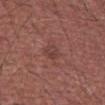The lesion was photographed on a routine skin check and not biopsied; there is no pathology result.
The subject is a male approximately 65 years of age.
Automated image analysis of the tile measured a within-lesion color-variation index near 3.5/10.
Captured under white-light illumination.
The lesion's longest dimension is about 2.5 mm.
A 15 mm close-up extracted from a 3D total-body photography capture.
The lesion is on the abdomen.